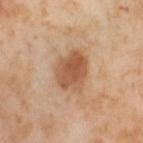Part of a total-body skin-imaging series; this lesion was reviewed on a skin check and was not flagged for biopsy.